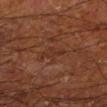Case summary:
– follow-up: total-body-photography surveillance lesion; no biopsy
– imaging modality: total-body-photography crop, ~15 mm field of view
– subject: male, approximately 65 years of age
– TBP lesion metrics: a lesion area of about 3 mm², a shape eccentricity near 0.9, and a shape-asymmetry score of about 0.4 (0 = symmetric); a detector confidence of about 95 out of 100 that the crop contains a lesion
– body site: the left lower leg